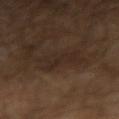follow-up=imaged on a skin check; not biopsied